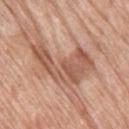No biopsy was performed on this lesion — it was imaged during a full skin examination and was not determined to be concerning. From the right thigh. About 8 mm across. The patient is a female about 75 years old. A close-up tile cropped from a whole-body skin photograph, about 15 mm across. This is a white-light tile.